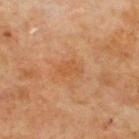This lesion was catalogued during total-body skin photography and was not selected for biopsy.
This image is a 15 mm lesion crop taken from a total-body photograph.
On the upper back.
A male patient, aged 68–72.